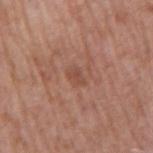No biopsy was performed on this lesion — it was imaged during a full skin examination and was not determined to be concerning. The lesion is on the right upper arm. A roughly 15 mm field-of-view crop from a total-body skin photograph. The patient is a male approximately 70 years of age. Automated image analysis of the tile measured a lesion color around L≈49 a*≈23 b*≈28 in CIELAB and a normalized lesion–skin contrast near 5. The analysis additionally found a border-irregularity rating of about 3/10, a color-variation rating of about 1/10, and a peripheral color-asymmetry measure near 0.5. It also reported lesion-presence confidence of about 100/100. This is a white-light tile. The recorded lesion diameter is about 2.5 mm.